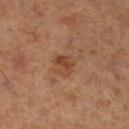Assessment: The lesion was tiled from a total-body skin photograph and was not biopsied. Clinical summary: A male subject about 70 years old. From the leg. The total-body-photography lesion software estimated an area of roughly 5 mm², a shape eccentricity near 0.65, and two-axis asymmetry of about 0.25. It also reported a mean CIELAB color near L≈35 a*≈18 b*≈27, about 7 CIELAB-L* units darker than the surrounding skin, and a normalized lesion–skin contrast near 6.5. It also reported an automated nevus-likeness rating near 45 out of 100. A 15 mm crop from a total-body photograph taken for skin-cancer surveillance.